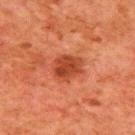Clinical impression: Imaged during a routine full-body skin examination; the lesion was not biopsied and no histopathology is available. Acquisition and patient details: Automated tile analysis of the lesion measured an area of roughly 9.5 mm², an eccentricity of roughly 0.55, and a symmetry-axis asymmetry near 0.25. The software also gave a mean CIELAB color near L≈36 a*≈28 b*≈32, roughly 9 lightness units darker than nearby skin, and a normalized border contrast of about 8. Cropped from a total-body skin-imaging series; the visible field is about 15 mm. Measured at roughly 3.5 mm in maximum diameter. The lesion is on the upper back. A male patient aged approximately 80.